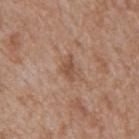{"biopsy_status": "not biopsied; imaged during a skin examination", "site": "mid back", "patient": {"sex": "male", "age_approx": 65}, "image": {"source": "total-body photography crop", "field_of_view_mm": 15}}